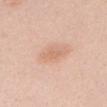notes: imaged on a skin check; not biopsied
patient: female, approximately 20 years of age
image: total-body-photography crop, ~15 mm field of view
body site: the head or neck